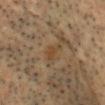Impression: The lesion was photographed on a routine skin check and not biopsied; there is no pathology result. Acquisition and patient details: The lesion's longest dimension is about 2.5 mm. A 15 mm crop from a total-body photograph taken for skin-cancer surveillance. Located on the head or neck. A male subject aged around 45. Imaged with cross-polarized lighting.